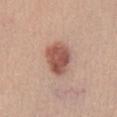Image and clinical context:
A region of skin cropped from a whole-body photographic capture, roughly 15 mm wide. The patient is a female aged 38–42. The lesion is located on the chest.